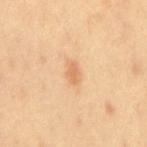Assessment:
This lesion was catalogued during total-body skin photography and was not selected for biopsy.
Context:
This image is a 15 mm lesion crop taken from a total-body photograph. Located on the mid back. The patient is a male aged around 40. An algorithmic analysis of the crop reported an average lesion color of about L≈69 a*≈22 b*≈40 (CIELAB) and a lesion-to-skin contrast of about 6 (normalized; higher = more distinct). It also reported a border-irregularity rating of about 2.5/10, a color-variation rating of about 1/10, and peripheral color asymmetry of about 0.5. It also reported a nevus-likeness score of about 30/100 and lesion-presence confidence of about 100/100. This is a cross-polarized tile.